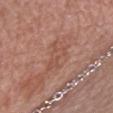Q: Was a biopsy performed?
A: imaged on a skin check; not biopsied
Q: What is the imaging modality?
A: ~15 mm tile from a whole-body skin photo
Q: How was the tile lit?
A: white-light
Q: What are the patient's age and sex?
A: female, approximately 60 years of age
Q: Lesion location?
A: the chest